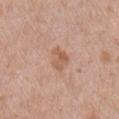The lesion was tiled from a total-body skin photograph and was not biopsied. The patient is a male aged approximately 50. Cropped from a whole-body photographic skin survey; the tile spans about 15 mm. Captured under white-light illumination. From the right upper arm. The lesion's longest dimension is about 3 mm.